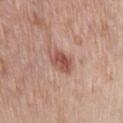| feature | finding |
|---|---|
| follow-up | catalogued during a skin exam; not biopsied |
| image-analysis metrics | a footprint of about 6 mm² and two-axis asymmetry of about 0.2; a lesion color around L≈53 a*≈24 b*≈27 in CIELAB and a lesion-to-skin contrast of about 8 (normalized; higher = more distinct) |
| subject | male, approximately 70 years of age |
| lesion size | about 3.5 mm |
| location | the chest |
| image | ~15 mm crop, total-body skin-cancer survey |
| lighting | white-light illumination |